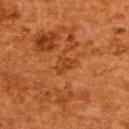Q: Is there a histopathology result?
A: catalogued during a skin exam; not biopsied
Q: What is the imaging modality?
A: 15 mm crop, total-body photography
Q: What is the anatomic site?
A: the back
Q: What are the patient's age and sex?
A: female, in their 50s